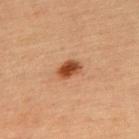Recorded during total-body skin imaging; not selected for excision or biopsy.
The patient is a female aged around 65.
A 15 mm close-up tile from a total-body photography series done for melanoma screening.
The lesion is on the upper back.
Imaged with cross-polarized lighting.